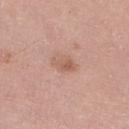No biopsy was performed on this lesion — it was imaged during a full skin examination and was not determined to be concerning. Captured under white-light illumination. The patient is a female aged approximately 65. Cropped from a whole-body photographic skin survey; the tile spans about 15 mm. The recorded lesion diameter is about 3 mm. Located on the right lower leg.